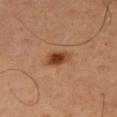No biopsy was performed on this lesion — it was imaged during a full skin examination and was not determined to be concerning. Captured under cross-polarized illumination. A male patient, aged 48–52. The lesion is located on the right thigh. This image is a 15 mm lesion crop taken from a total-body photograph. Approximately 3 mm at its widest.